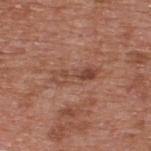This lesion was catalogued during total-body skin photography and was not selected for biopsy.
Captured under white-light illumination.
A 15 mm crop from a total-body photograph taken for skin-cancer surveillance.
A male patient, aged approximately 65.
Measured at roughly 5 mm in maximum diameter.
The lesion is on the upper back.